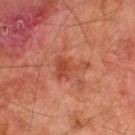Captured during whole-body skin photography for melanoma surveillance; the lesion was not biopsied.
This image is a 15 mm lesion crop taken from a total-body photograph.
The lesion is located on the right thigh.
Automated tile analysis of the lesion measured a normalized border contrast of about 6.5. It also reported an automated nevus-likeness rating near 0 out of 100.
Longest diameter approximately 4 mm.
A male subject, approximately 70 years of age.
This is a cross-polarized tile.